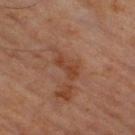Assessment:
Part of a total-body skin-imaging series; this lesion was reviewed on a skin check and was not flagged for biopsy.
Image and clinical context:
The tile uses cross-polarized illumination. Automated tile analysis of the lesion measured a lesion color around L≈38 a*≈23 b*≈30 in CIELAB, roughly 7 lightness units darker than nearby skin, and a normalized lesion–skin contrast near 7. It also reported a border-irregularity rating of about 6/10, a color-variation rating of about 0/10, and radial color variation of about 0. A 15 mm close-up tile from a total-body photography series done for melanoma screening. The lesion's longest dimension is about 3 mm. A male subject aged 58 to 62. The lesion is on the right thigh.